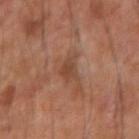The lesion was tiled from a total-body skin photograph and was not biopsied.
The lesion's longest dimension is about 3 mm.
The subject is a male roughly 60 years of age.
Captured under cross-polarized illumination.
Automated tile analysis of the lesion measured a footprint of about 5 mm², an eccentricity of roughly 0.8, and a symmetry-axis asymmetry near 0.4. And it measured a nevus-likeness score of about 0/100 and lesion-presence confidence of about 100/100.
The lesion is located on the arm.
A 15 mm crop from a total-body photograph taken for skin-cancer surveillance.